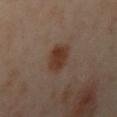<case>
<biopsy_status>not biopsied; imaged during a skin examination</biopsy_status>
<site>left arm</site>
<patient>
  <sex>female</sex>
  <age_approx>40</age_approx>
</patient>
<image>
  <source>total-body photography crop</source>
  <field_of_view_mm>15</field_of_view_mm>
</image>
</case>